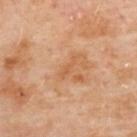Q: What kind of image is this?
A: ~15 mm tile from a whole-body skin photo
Q: Who is the patient?
A: female, aged around 60
Q: How large is the lesion?
A: ≈3 mm
Q: How was the tile lit?
A: cross-polarized
Q: Automated lesion metrics?
A: border irregularity of about 4.5 on a 0–10 scale, internal color variation of about 0 on a 0–10 scale, and radial color variation of about 0
Q: Lesion location?
A: the upper back